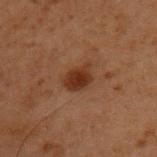Clinical impression: No biopsy was performed on this lesion — it was imaged during a full skin examination and was not determined to be concerning. Acquisition and patient details: Captured under cross-polarized illumination. From the left upper arm. A 15 mm crop from a total-body photograph taken for skin-cancer surveillance. The lesion's longest dimension is about 3.5 mm. The total-body-photography lesion software estimated a lesion area of about 6 mm² and an outline eccentricity of about 0.75 (0 = round, 1 = elongated). The software also gave a lesion color around L≈25 a*≈19 b*≈25 in CIELAB, roughly 8 lightness units darker than nearby skin, and a normalized lesion–skin contrast near 9.5. The subject is a male roughly 60 years of age.